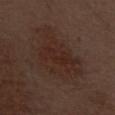{"biopsy_status": "not biopsied; imaged during a skin examination", "lighting": "white-light", "lesion_size": {"long_diameter_mm_approx": 8.0}, "patient": {"sex": "male", "age_approx": 70}, "image": {"source": "total-body photography crop", "field_of_view_mm": 15}, "site": "front of the torso", "automated_metrics": {"eccentricity": 0.8, "shape_asymmetry": 0.35, "vs_skin_darker_L": 5.0}}